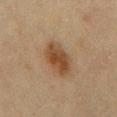This lesion was catalogued during total-body skin photography and was not selected for biopsy. The total-body-photography lesion software estimated an average lesion color of about L≈37 a*≈16 b*≈28 (CIELAB), roughly 9 lightness units darker than nearby skin, and a normalized border contrast of about 9. It also reported a border-irregularity rating of about 2/10, internal color variation of about 3.5 on a 0–10 scale, and a peripheral color-asymmetry measure near 1. A close-up tile cropped from a whole-body skin photograph, about 15 mm across. The lesion is on the chest. Captured under cross-polarized illumination. Measured at roughly 4.5 mm in maximum diameter. A male subject, aged approximately 70.